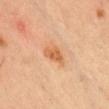- follow-up — catalogued during a skin exam; not biopsied
- patient — male, roughly 35 years of age
- location — the chest
- acquisition — ~15 mm tile from a whole-body skin photo
- illumination — cross-polarized illumination